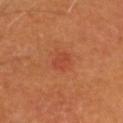* follow-up · catalogued during a skin exam; not biopsied
* body site · the head or neck
* lesion size · about 3 mm
* patient · male, approximately 60 years of age
* image source · total-body-photography crop, ~15 mm field of view
* lighting · cross-polarized